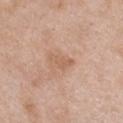biopsy_status: not biopsied; imaged during a skin examination
automated_metrics:
  area_mm2_approx: 3.5
  shape_asymmetry: 0.4
site: chest
patient:
  sex: female
  age_approx: 40
lesion_size:
  long_diameter_mm_approx: 3.0
lighting: white-light
image:
  source: total-body photography crop
  field_of_view_mm: 15